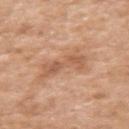Clinical impression: Imaged during a routine full-body skin examination; the lesion was not biopsied and no histopathology is available. Image and clinical context: A 15 mm close-up extracted from a 3D total-body photography capture. From the left upper arm. A male patient aged approximately 60. Automated image analysis of the tile measured a mean CIELAB color near L≈58 a*≈22 b*≈34, roughly 9 lightness units darker than nearby skin, and a normalized lesion–skin contrast near 6. The software also gave border irregularity of about 6 on a 0–10 scale, internal color variation of about 4 on a 0–10 scale, and peripheral color asymmetry of about 1.5. This is a white-light tile. The recorded lesion diameter is about 5.5 mm.